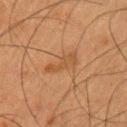- notes: imaged on a skin check; not biopsied
- subject: male, aged approximately 50
- location: the left upper arm
- acquisition: total-body-photography crop, ~15 mm field of view
- diameter: ≈3.5 mm